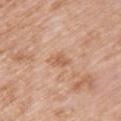Captured during whole-body skin photography for melanoma surveillance; the lesion was not biopsied.
The lesion's longest dimension is about 2.5 mm.
Automated image analysis of the tile measured a nevus-likeness score of about 0/100.
Captured under white-light illumination.
A 15 mm close-up tile from a total-body photography series done for melanoma screening.
On the left upper arm.
A female subject, aged 68–72.